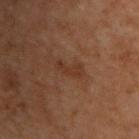The lesion was photographed on a routine skin check and not biopsied; there is no pathology result. The lesion's longest dimension is about 3.5 mm. A 15 mm close-up extracted from a 3D total-body photography capture. A male subject aged 68–72. Captured under cross-polarized illumination. Located on the upper back. Automated image analysis of the tile measured a border-irregularity rating of about 6.5/10 and a within-lesion color-variation index near 0/10. And it measured a classifier nevus-likeness of about 0/100 and a lesion-detection confidence of about 95/100.